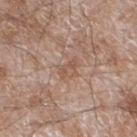Case summary:
• image: ~15 mm crop, total-body skin-cancer survey
• location: the left lower leg
• patient: male, in their 60s